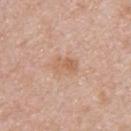notes: total-body-photography surveillance lesion; no biopsy | automated lesion analysis: a lesion color around L≈61 a*≈21 b*≈32 in CIELAB and a normalized border contrast of about 6 | anatomic site: the upper back | acquisition: 15 mm crop, total-body photography | tile lighting: white-light illumination | patient: male, in their 50s.